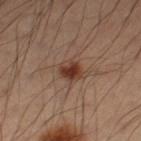Part of a total-body skin-imaging series; this lesion was reviewed on a skin check and was not flagged for biopsy. Located on the leg. A male subject, in their 50s. Cropped from a whole-body photographic skin survey; the tile spans about 15 mm. The tile uses cross-polarized illumination. Measured at roughly 2.5 mm in maximum diameter.